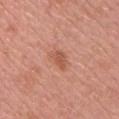<case>
  <biopsy_status>not biopsied; imaged during a skin examination</biopsy_status>
  <image>
    <source>total-body photography crop</source>
    <field_of_view_mm>15</field_of_view_mm>
  </image>
  <patient>
    <sex>male</sex>
    <age_approx>55</age_approx>
  </patient>
  <lesion_size>
    <long_diameter_mm_approx>2.5</long_diameter_mm_approx>
  </lesion_size>
  <site>chest</site>
</case>